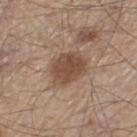Notes:
- body site — the left thigh
- lighting — white-light illumination
- TBP lesion metrics — a nevus-likeness score of about 90/100 and lesion-presence confidence of about 100/100
- patient — male, aged 58–62
- acquisition — ~15 mm tile from a whole-body skin photo
- size — about 4.5 mm